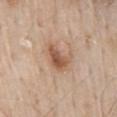Q: Lesion location?
A: the mid back
Q: What are the patient's age and sex?
A: male, aged 58 to 62
Q: Illumination type?
A: white-light illumination
Q: What kind of image is this?
A: 15 mm crop, total-body photography
Q: What is the lesion's diameter?
A: ~3.5 mm (longest diameter)
Q: Automated lesion metrics?
A: an outline eccentricity of about 0.7 (0 = round, 1 = elongated) and a symmetry-axis asymmetry near 0.35; border irregularity of about 3.5 on a 0–10 scale, internal color variation of about 5 on a 0–10 scale, and radial color variation of about 1.5; lesion-presence confidence of about 100/100